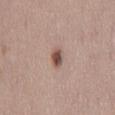{
  "biopsy_status": "not biopsied; imaged during a skin examination",
  "lesion_size": {
    "long_diameter_mm_approx": 3.0
  },
  "automated_metrics": {
    "shape_asymmetry": 0.3,
    "border_irregularity_0_10": 2.5,
    "color_variation_0_10": 5.0
  },
  "patient": {
    "sex": "female",
    "age_approx": 50
  },
  "site": "mid back",
  "image": {
    "source": "total-body photography crop",
    "field_of_view_mm": 15
  },
  "lighting": "white-light"
}